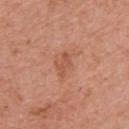workup: no biopsy performed (imaged during a skin exam)
subject: female, aged 58–62
site: the arm
imaging modality: ~15 mm tile from a whole-body skin photo
tile lighting: white-light
diameter: about 3 mm
automated lesion analysis: an area of roughly 2.5 mm²; an average lesion color of about L≈54 a*≈26 b*≈33 (CIELAB) and a lesion-to-skin contrast of about 5.5 (normalized; higher = more distinct); a border-irregularity index near 6.5/10, internal color variation of about 0 on a 0–10 scale, and radial color variation of about 0; an automated nevus-likeness rating near 0 out of 100